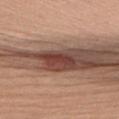Impression: No biopsy was performed on this lesion — it was imaged during a full skin examination and was not determined to be concerning. Context: A roughly 15 mm field-of-view crop from a total-body skin photograph. The lesion is on the right upper arm. A male subject approximately 55 years of age. The recorded lesion diameter is about 4.5 mm. The lesion-visualizer software estimated a footprint of about 5.5 mm², an eccentricity of roughly 0.95, and a symmetry-axis asymmetry near 0.35. It also reported a normalized lesion–skin contrast near 11.5. It also reported an automated nevus-likeness rating near 100 out of 100. Imaged with white-light lighting.